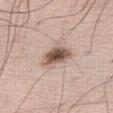Case summary:
– lighting — white-light
– acquisition — total-body-photography crop, ~15 mm field of view
– TBP lesion metrics — an area of roughly 9 mm² and a symmetry-axis asymmetry near 0.3; border irregularity of about 3 on a 0–10 scale, a within-lesion color-variation index near 6.5/10, and peripheral color asymmetry of about 1.5; a classifier nevus-likeness of about 95/100 and lesion-presence confidence of about 100/100
– anatomic site — the right thigh
– subject — male, aged 68 to 72